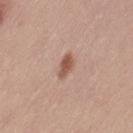<tbp_lesion>
  <biopsy_status>not biopsied; imaged during a skin examination</biopsy_status>
  <image>
    <source>total-body photography crop</source>
    <field_of_view_mm>15</field_of_view_mm>
  </image>
  <lighting>white-light</lighting>
  <site>left thigh</site>
  <patient>
    <sex>female</sex>
    <age_approx>35</age_approx>
  </patient>
  <lesion_size>
    <long_diameter_mm_approx>3.0</long_diameter_mm_approx>
  </lesion_size>
</tbp_lesion>